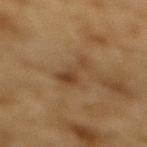Captured during whole-body skin photography for melanoma surveillance; the lesion was not biopsied. The lesion-visualizer software estimated a footprint of about 6.5 mm² and a shape eccentricity near 0.9. And it measured border irregularity of about 6.5 on a 0–10 scale, a color-variation rating of about 2.5/10, and peripheral color asymmetry of about 0.5. The analysis additionally found an automated nevus-likeness rating near 0 out of 100 and a detector confidence of about 100 out of 100 that the crop contains a lesion. A male patient, about 85 years old or older. Located on the mid back. A 15 mm close-up tile from a total-body photography series done for melanoma screening.